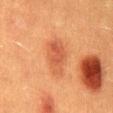Q: Was a biopsy performed?
A: imaged on a skin check; not biopsied
Q: How large is the lesion?
A: about 4.5 mm
Q: Patient demographics?
A: male, in their 40s
Q: What is the anatomic site?
A: the mid back
Q: What is the imaging modality?
A: ~15 mm tile from a whole-body skin photo